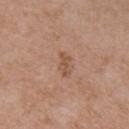Q: Was this lesion biopsied?
A: no biopsy performed (imaged during a skin exam)
Q: What is the lesion's diameter?
A: ~3 mm (longest diameter)
Q: How was this image acquired?
A: 15 mm crop, total-body photography
Q: Patient demographics?
A: male, roughly 65 years of age
Q: What lighting was used for the tile?
A: white-light illumination
Q: Lesion location?
A: the chest